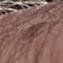<lesion>
<biopsy_status>not biopsied; imaged during a skin examination</biopsy_status>
<lighting>white-light</lighting>
<patient>
  <sex>male</sex>
  <age_approx>75</age_approx>
</patient>
<image>
  <source>total-body photography crop</source>
  <field_of_view_mm>15</field_of_view_mm>
</image>
<site>left forearm</site>
<lesion_size>
  <long_diameter_mm_approx>5.5</long_diameter_mm_approx>
</lesion_size>
<automated_metrics>
  <vs_skin_darker_L>8.0</vs_skin_darker_L>
  <border_irregularity_0_10>3.5</border_irregularity_0_10>
  <color_variation_0_10>2.5</color_variation_0_10>
  <peripheral_color_asymmetry>1.0</peripheral_color_asymmetry>
</automated_metrics>
</lesion>